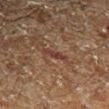Assessment: This lesion was catalogued during total-body skin photography and was not selected for biopsy. Acquisition and patient details: A male subject, about 60 years old. Measured at roughly 3 mm in maximum diameter. A close-up tile cropped from a whole-body skin photograph, about 15 mm across. Imaged with cross-polarized lighting. The lesion is on the right lower leg.